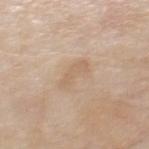{
  "biopsy_status": "not biopsied; imaged during a skin examination",
  "patient": {
    "sex": "male",
    "age_approx": "85+"
  },
  "image": {
    "source": "total-body photography crop",
    "field_of_view_mm": 15
  },
  "site": "upper back"
}